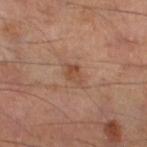The lesion was photographed on a routine skin check and not biopsied; there is no pathology result.
A male subject, in their mid- to late 50s.
A 15 mm close-up extracted from a 3D total-body photography capture.
From the leg.
The total-body-photography lesion software estimated a mean CIELAB color near L≈44 a*≈20 b*≈28, a lesion–skin lightness drop of about 7, and a normalized border contrast of about 6. The analysis additionally found a border-irregularity index near 2.5/10, internal color variation of about 2.5 on a 0–10 scale, and radial color variation of about 1. The analysis additionally found an automated nevus-likeness rating near 25 out of 100.
The tile uses cross-polarized illumination.